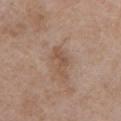{
  "image": {
    "source": "total-body photography crop",
    "field_of_view_mm": 15
  },
  "patient": {
    "sex": "male",
    "age_approx": 50
  },
  "site": "front of the torso",
  "lesion_size": {
    "long_diameter_mm_approx": 4.0
  },
  "lighting": "white-light"
}